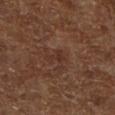{"biopsy_status": "not biopsied; imaged during a skin examination", "automated_metrics": {"shape_asymmetry": 0.5, "vs_skin_darker_L": 5.0, "border_irregularity_0_10": 5.0, "color_variation_0_10": 0.0, "peripheral_color_asymmetry": 0.0, "nevus_likeness_0_100": 0, "lesion_detection_confidence_0_100": 100}, "image": {"source": "total-body photography crop", "field_of_view_mm": 15}, "lesion_size": {"long_diameter_mm_approx": 2.5}, "site": "right lower leg", "patient": {"age_approx": 65}, "lighting": "cross-polarized"}